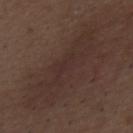| feature | finding |
|---|---|
| biopsy status | no biopsy performed (imaged during a skin exam) |
| patient | male, about 50 years old |
| acquisition | total-body-photography crop, ~15 mm field of view |
| lesion size | ≈10.5 mm |
| anatomic site | the chest |
| lighting | white-light illumination |